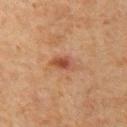biopsy_status: not biopsied; imaged during a skin examination
lighting: cross-polarized
site: chest
patient:
  sex: male
  age_approx: 60
image:
  source: total-body photography crop
  field_of_view_mm: 15
lesion_size:
  long_diameter_mm_approx: 3.0